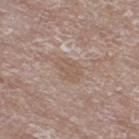| field | value |
|---|---|
| notes | catalogued during a skin exam; not biopsied |
| subject | female, in their mid- to late 70s |
| tile lighting | white-light |
| anatomic site | the right thigh |
| automated lesion analysis | an average lesion color of about L≈55 a*≈16 b*≈26 (CIELAB), a lesion–skin lightness drop of about 6, and a lesion-to-skin contrast of about 5.5 (normalized; higher = more distinct) |
| imaging modality | 15 mm crop, total-body photography |
| lesion diameter | ~3 mm (longest diameter) |